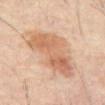Captured during whole-body skin photography for melanoma surveillance; the lesion was not biopsied. About 7.5 mm across. The tile uses cross-polarized illumination. The subject is a male about 60 years old. The lesion is on the abdomen. A lesion tile, about 15 mm wide, cut from a 3D total-body photograph. Automated tile analysis of the lesion measured an average lesion color of about L≈59 a*≈20 b*≈31 (CIELAB), a lesion–skin lightness drop of about 9, and a normalized border contrast of about 6.5. And it measured border irregularity of about 4.5 on a 0–10 scale and peripheral color asymmetry of about 1.5. The software also gave an automated nevus-likeness rating near 10 out of 100 and a lesion-detection confidence of about 100/100.